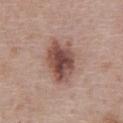Case summary:
• follow-up · imaged on a skin check; not biopsied
• size · ~5.5 mm (longest diameter)
• lighting · white-light
• automated metrics · about 15 CIELAB-L* units darker than the surrounding skin and a normalized lesion–skin contrast near 10.5
• body site · the abdomen
• patient · male, in their 60s
• imaging modality · total-body-photography crop, ~15 mm field of view